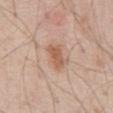Assessment: The lesion was tiled from a total-body skin photograph and was not biopsied. Background: A lesion tile, about 15 mm wide, cut from a 3D total-body photograph. A male patient roughly 70 years of age. From the abdomen. The tile uses white-light illumination. The total-body-photography lesion software estimated two-axis asymmetry of about 0.25. The analysis additionally found a border-irregularity index near 3/10, a within-lesion color-variation index near 3/10, and peripheral color asymmetry of about 1. The software also gave a lesion-detection confidence of about 100/100. Longest diameter approximately 3.5 mm.